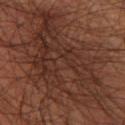Part of a total-body skin-imaging series; this lesion was reviewed on a skin check and was not flagged for biopsy. On the leg. Captured under cross-polarized illumination. A male patient approximately 60 years of age. Measured at roughly 14 mm in maximum diameter. A 15 mm close-up tile from a total-body photography series done for melanoma screening.